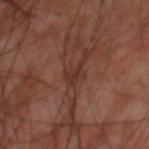<lesion>
  <biopsy_status>not biopsied; imaged during a skin examination</biopsy_status>
  <image>
    <source>total-body photography crop</source>
    <field_of_view_mm>15</field_of_view_mm>
  </image>
  <patient>
    <sex>male</sex>
    <age_approx>60</age_approx>
  </patient>
  <site>back</site>
</lesion>